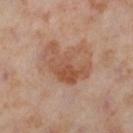follow-up = total-body-photography surveillance lesion; no biopsy
lesion diameter = ~6.5 mm (longest diameter)
subject = female, aged 53 to 57
tile lighting = cross-polarized illumination
imaging modality = total-body-photography crop, ~15 mm field of view
body site = the left lower leg